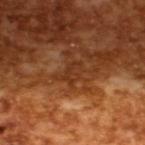Imaged during a routine full-body skin examination; the lesion was not biopsied and no histopathology is available. A male patient, aged around 65. A 15 mm close-up extracted from a 3D total-body photography capture. This is a cross-polarized tile. The lesion-visualizer software estimated a lesion area of about 3.5 mm² and a shape-asymmetry score of about 0.5 (0 = symmetric). And it measured a border-irregularity rating of about 6/10 and a color-variation rating of about 0/10. It also reported a nevus-likeness score of about 0/100 and a lesion-detection confidence of about 90/100. The recorded lesion diameter is about 2.5 mm.